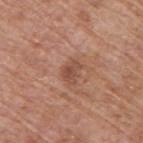This lesion was catalogued during total-body skin photography and was not selected for biopsy. Longest diameter approximately 2.5 mm. On the upper back. The subject is a male in their mid-60s. A 15 mm close-up tile from a total-body photography series done for melanoma screening.